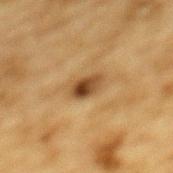biopsy status=total-body-photography surveillance lesion; no biopsy
site=the mid back
subject=male, roughly 85 years of age or older
image source=15 mm crop, total-body photography